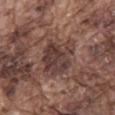Impression: The lesion was tiled from a total-body skin photograph and was not biopsied. Background: The total-body-photography lesion software estimated a lesion-to-skin contrast of about 8.5 (normalized; higher = more distinct). And it measured a classifier nevus-likeness of about 5/100. Captured under white-light illumination. The subject is a male about 75 years old. Cropped from a whole-body photographic skin survey; the tile spans about 15 mm. The lesion's longest dimension is about 4.5 mm. On the mid back.